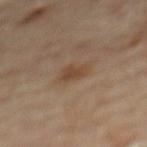Impression:
Recorded during total-body skin imaging; not selected for excision or biopsy.
Clinical summary:
The lesion is on the mid back. A 15 mm close-up extracted from a 3D total-body photography capture. About 3 mm across. The subject is a male approximately 85 years of age or older.